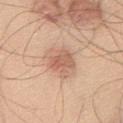workup: imaged on a skin check; not biopsied
location: the chest
lighting: white-light
patient: male, aged approximately 45
automated lesion analysis: a lesion area of about 12 mm², an outline eccentricity of about 0.6 (0 = round, 1 = elongated), and a symmetry-axis asymmetry near 0.2; an average lesion color of about L≈63 a*≈20 b*≈31 (CIELAB), about 10 CIELAB-L* units darker than the surrounding skin, and a normalized border contrast of about 6.5; a detector confidence of about 100 out of 100 that the crop contains a lesion
image source: ~15 mm crop, total-body skin-cancer survey